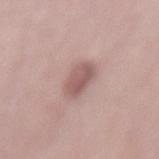| feature | finding |
|---|---|
| notes | catalogued during a skin exam; not biopsied |
| patient | female, roughly 65 years of age |
| anatomic site | the mid back |
| acquisition | 15 mm crop, total-body photography |